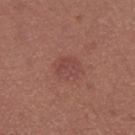Findings:
– follow-up · catalogued during a skin exam; not biopsied
– patient · female, in their mid-30s
– acquisition · ~15 mm tile from a whole-body skin photo
– body site · the leg